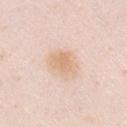The tile uses white-light illumination. A female subject aged approximately 30. A 15 mm crop from a total-body photograph taken for skin-cancer surveillance. The lesion is on the front of the torso. Measured at roughly 4 mm in maximum diameter.